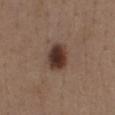No biopsy was performed on this lesion — it was imaged during a full skin examination and was not determined to be concerning. A female patient about 40 years old. The tile uses white-light illumination. Measured at roughly 4 mm in maximum diameter. A 15 mm close-up tile from a total-body photography series done for melanoma screening. The lesion is located on the chest.